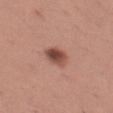No biopsy was performed on this lesion — it was imaged during a full skin examination and was not determined to be concerning. The lesion is located on the left thigh. A 15 mm crop from a total-body photograph taken for skin-cancer surveillance. Imaged with white-light lighting. A female subject aged 28 to 32.